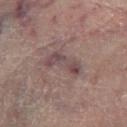Clinical impression: This lesion was catalogued during total-body skin photography and was not selected for biopsy. Image and clinical context: The lesion's longest dimension is about 4.5 mm. The tile uses cross-polarized illumination. The patient is a male aged 63 to 67. The lesion is located on the left lower leg. A roughly 15 mm field-of-view crop from a total-body skin photograph.